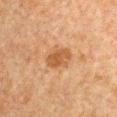Q: Is there a histopathology result?
A: no biopsy performed (imaged during a skin exam)